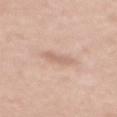biopsy_status: not biopsied; imaged during a skin examination
image:
  source: total-body photography crop
  field_of_view_mm: 15
site: upper back
patient:
  sex: female
  age_approx: 35
lighting: white-light
automated_metrics:
  area_mm2_approx: 3.0
  eccentricity: 0.9
  cielab_L: 64
  cielab_a: 19
  cielab_b: 28
  vs_skin_darker_L: 8.0
  vs_skin_contrast_norm: 5.0
  border_irregularity_0_10: 3.5
  color_variation_0_10: 0.0
  peripheral_color_asymmetry: 0.0
  nevus_likeness_0_100: 0
  lesion_detection_confidence_0_100: 100
lesion_size:
  long_diameter_mm_approx: 3.0